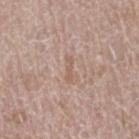Findings:
* notes: total-body-photography surveillance lesion; no biopsy
* illumination: white-light
* patient: female, aged 58–62
* size: about 3 mm
* automated lesion analysis: an average lesion color of about L≈58 a*≈18 b*≈26 (CIELAB) and a normalized border contrast of about 5; a lesion-detection confidence of about 80/100
* site: the leg
* image source: ~15 mm crop, total-body skin-cancer survey